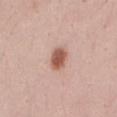This is a white-light tile. A close-up tile cropped from a whole-body skin photograph, about 15 mm across. The lesion-visualizer software estimated a lesion area of about 5 mm², an outline eccentricity of about 0.7 (0 = round, 1 = elongated), and a shape-asymmetry score of about 0.15 (0 = symmetric). The analysis additionally found border irregularity of about 1.5 on a 0–10 scale, a color-variation rating of about 3/10, and peripheral color asymmetry of about 1. Measured at roughly 3 mm in maximum diameter. The subject is a female aged 23 to 27. From the abdomen.